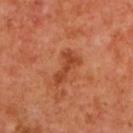Q: Is there a histopathology result?
A: imaged on a skin check; not biopsied
Q: How large is the lesion?
A: about 4 mm
Q: Where on the body is the lesion?
A: the upper back
Q: What lighting was used for the tile?
A: cross-polarized illumination
Q: Patient demographics?
A: female, aged approximately 40
Q: How was this image acquired?
A: 15 mm crop, total-body photography